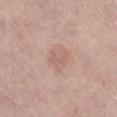{
  "biopsy_status": "not biopsied; imaged during a skin examination",
  "site": "left lower leg",
  "patient": {
    "sex": "female",
    "age_approx": 65
  },
  "image": {
    "source": "total-body photography crop",
    "field_of_view_mm": 15
  },
  "lesion_size": {
    "long_diameter_mm_approx": 3.0
  }
}